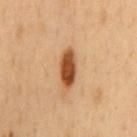The lesion was photographed on a routine skin check and not biopsied; there is no pathology result.
Located on the back.
The lesion-visualizer software estimated an eccentricity of roughly 0.9 and a shape-asymmetry score of about 0.15 (0 = symmetric). The software also gave roughly 18 lightness units darker than nearby skin.
This is a cross-polarized tile.
Approximately 4.5 mm at its widest.
A 15 mm close-up extracted from a 3D total-body photography capture.
A male subject aged approximately 50.